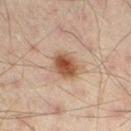Q: Was this lesion biopsied?
A: total-body-photography surveillance lesion; no biopsy
Q: What are the patient's age and sex?
A: male, in their mid-40s
Q: What did automated image analysis measure?
A: a mean CIELAB color near L≈46 a*≈17 b*≈27 and a lesion–skin lightness drop of about 11; lesion-presence confidence of about 100/100
Q: What kind of image is this?
A: 15 mm crop, total-body photography
Q: Lesion location?
A: the left lower leg
Q: Illumination type?
A: cross-polarized
Q: What is the lesion's diameter?
A: ≈4 mm